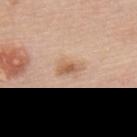| key | value |
|---|---|
| follow-up | total-body-photography surveillance lesion; no biopsy |
| acquisition | 15 mm crop, total-body photography |
| location | the upper back |
| patient | female, approximately 65 years of age |